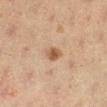{"biopsy_status": "not biopsied; imaged during a skin examination", "site": "right lower leg", "lesion_size": {"long_diameter_mm_approx": 2.5}, "image": {"source": "total-body photography crop", "field_of_view_mm": 15}, "patient": {"sex": "female", "age_approx": 20}}